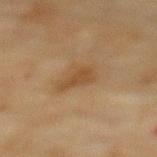Captured during whole-body skin photography for melanoma surveillance; the lesion was not biopsied.
A female subject, roughly 80 years of age.
Cropped from a total-body skin-imaging series; the visible field is about 15 mm.
The lesion is on the upper back.
Longest diameter approximately 3 mm.